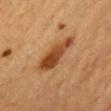<tbp_lesion>
  <biopsy_status>not biopsied; imaged during a skin examination</biopsy_status>
  <lighting>cross-polarized</lighting>
  <automated_metrics>
    <border_irregularity_0_10>4.0</border_irregularity_0_10>
    <color_variation_0_10>5.0</color_variation_0_10>
    <peripheral_color_asymmetry>1.5</peripheral_color_asymmetry>
    <nevus_likeness_0_100>95</nevus_likeness_0_100>
    <lesion_detection_confidence_0_100>100</lesion_detection_confidence_0_100>
  </automated_metrics>
  <patient>
    <sex>female</sex>
    <age_approx>40</age_approx>
  </patient>
  <lesion_size>
    <long_diameter_mm_approx>6.0</long_diameter_mm_approx>
  </lesion_size>
  <image>
    <source>total-body photography crop</source>
    <field_of_view_mm>15</field_of_view_mm>
  </image>
  <site>head or neck</site>
</tbp_lesion>